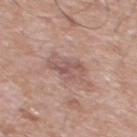Assessment: The lesion was tiled from a total-body skin photograph and was not biopsied. Context: A 15 mm close-up tile from a total-body photography series done for melanoma screening. The tile uses white-light illumination. From the chest. Measured at roughly 4 mm in maximum diameter. The subject is a male approximately 70 years of age. An algorithmic analysis of the crop reported a footprint of about 6.5 mm², a shape eccentricity near 0.8, and a symmetry-axis asymmetry near 0.35. The software also gave an average lesion color of about L≈54 a*≈20 b*≈23 (CIELAB), about 8 CIELAB-L* units darker than the surrounding skin, and a normalized border contrast of about 6.